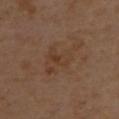<lesion>
<biopsy_status>not biopsied; imaged during a skin examination</biopsy_status>
<patient>
  <sex>male</sex>
  <age_approx>55</age_approx>
</patient>
<automated_metrics>
  <area_mm2_approx>10.0</area_mm2_approx>
  <eccentricity>0.8</eccentricity>
  <shape_asymmetry>0.5</shape_asymmetry>
  <cielab_L>38</cielab_L>
  <cielab_a>17</cielab_a>
  <cielab_b>29</cielab_b>
  <vs_skin_darker_L>5.0</vs_skin_darker_L>
  <border_irregularity_0_10>7.5</border_irregularity_0_10>
  <color_variation_0_10>4.0</color_variation_0_10>
  <peripheral_color_asymmetry>1.5</peripheral_color_asymmetry>
</automated_metrics>
<site>upper back</site>
<lesion_size>
  <long_diameter_mm_approx>6.0</long_diameter_mm_approx>
</lesion_size>
<lighting>cross-polarized</lighting>
<image>
  <source>total-body photography crop</source>
  <field_of_view_mm>15</field_of_view_mm>
</image>
</lesion>